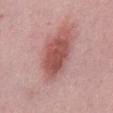No biopsy was performed on this lesion — it was imaged during a full skin examination and was not determined to be concerning. The lesion is located on the abdomen. Cropped from a total-body skin-imaging series; the visible field is about 15 mm. The recorded lesion diameter is about 5.5 mm. The patient is a male aged approximately 50.